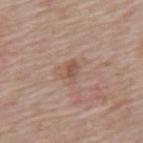Clinical impression: This lesion was catalogued during total-body skin photography and was not selected for biopsy.